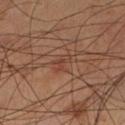notes: catalogued during a skin exam; not biopsied | anatomic site: the left lower leg | illumination: cross-polarized illumination | acquisition: 15 mm crop, total-body photography | subject: male, about 60 years old | image-analysis metrics: an area of roughly 3 mm² and an outline eccentricity of about 0.8 (0 = round, 1 = elongated); a detector confidence of about 90 out of 100 that the crop contains a lesion | size: ≈2.5 mm.